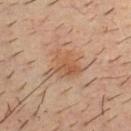Clinical impression:
The lesion was tiled from a total-body skin photograph and was not biopsied.
Context:
The lesion-visualizer software estimated roughly 7 lightness units darker than nearby skin. And it measured a border-irregularity index near 4.5/10, internal color variation of about 4 on a 0–10 scale, and a peripheral color-asymmetry measure near 1.5. The software also gave a classifier nevus-likeness of about 45/100 and lesion-presence confidence of about 100/100. Captured under cross-polarized illumination. Approximately 4.5 mm at its widest. A male subject, aged 33 to 37. From the chest. A 15 mm close-up extracted from a 3D total-body photography capture.